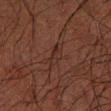Captured during whole-body skin photography for melanoma surveillance; the lesion was not biopsied. A 15 mm close-up tile from a total-body photography series done for melanoma screening. A male patient, aged approximately 60. Located on the left forearm. This is a cross-polarized tile. About 3 mm across.